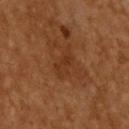The lesion was photographed on a routine skin check and not biopsied; there is no pathology result. The lesion's longest dimension is about 3 mm. The total-body-photography lesion software estimated roughly 5 lightness units darker than nearby skin and a normalized border contrast of about 5.5. It also reported lesion-presence confidence of about 100/100. This image is a 15 mm lesion crop taken from a total-body photograph. The tile uses cross-polarized illumination. On the upper back. The subject is a male aged 58 to 62.